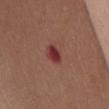Impression:
No biopsy was performed on this lesion — it was imaged during a full skin examination and was not determined to be concerning.
Context:
The lesion is located on the chest. A lesion tile, about 15 mm wide, cut from a 3D total-body photograph. An algorithmic analysis of the crop reported a lesion area of about 4.5 mm², an outline eccentricity of about 0.7 (0 = round, 1 = elongated), and a symmetry-axis asymmetry near 0.15. And it measured an average lesion color of about L≈37 a*≈29 b*≈22 (CIELAB), roughly 11 lightness units darker than nearby skin, and a normalized border contrast of about 10. It also reported a classifier nevus-likeness of about 0/100 and lesion-presence confidence of about 100/100. A female patient, in their mid-60s. This is a white-light tile. The lesion's longest dimension is about 2.5 mm.